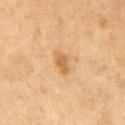  lesion_size:
    long_diameter_mm_approx: 2.5
  image:
    source: total-body photography crop
    field_of_view_mm: 15
  lighting: cross-polarized
  patient:
    sex: female
    age_approx: 55
  site: left thigh